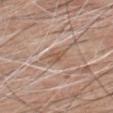Captured during whole-body skin photography for melanoma surveillance; the lesion was not biopsied.
Imaged with white-light lighting.
The recorded lesion diameter is about 2.5 mm.
From the chest.
A region of skin cropped from a whole-body photographic capture, roughly 15 mm wide.
A male subject, aged 58 to 62.